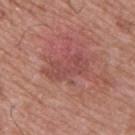Part of a total-body skin-imaging series; this lesion was reviewed on a skin check and was not flagged for biopsy. A male subject, aged around 65. About 5 mm across. Captured under white-light illumination. The lesion is on the upper back. A roughly 15 mm field-of-view crop from a total-body skin photograph.